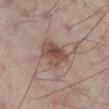Q: Where on the body is the lesion?
A: the left lower leg
Q: Who is the patient?
A: male, aged around 60
Q: What is the imaging modality?
A: ~15 mm crop, total-body skin-cancer survey
Q: What did automated image analysis measure?
A: roughly 10 lightness units darker than nearby skin and a normalized lesion–skin contrast near 8
Q: How was the tile lit?
A: white-light
Q: What is the lesion's diameter?
A: ≈4 mm